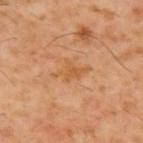The lesion was tiled from a total-body skin photograph and was not biopsied. A male subject, aged approximately 60. From the upper back. This image is a 15 mm lesion crop taken from a total-body photograph.